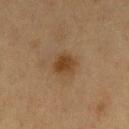Captured during whole-body skin photography for melanoma surveillance; the lesion was not biopsied.
Captured under cross-polarized illumination.
Located on the left thigh.
A female patient, about 40 years old.
The lesion's longest dimension is about 3 mm.
An algorithmic analysis of the crop reported an average lesion color of about L≈36 a*≈16 b*≈30 (CIELAB), roughly 8 lightness units darker than nearby skin, and a lesion-to-skin contrast of about 8 (normalized; higher = more distinct). The software also gave a lesion-detection confidence of about 100/100.
A 15 mm close-up tile from a total-body photography series done for melanoma screening.